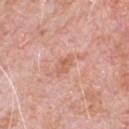workup: catalogued during a skin exam; not biopsied